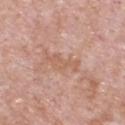Clinical impression: The lesion was tiled from a total-body skin photograph and was not biopsied. Context: The lesion's longest dimension is about 4.5 mm. Cropped from a total-body skin-imaging series; the visible field is about 15 mm. The total-body-photography lesion software estimated a lesion area of about 5 mm² and a symmetry-axis asymmetry near 0.5. The software also gave border irregularity of about 7 on a 0–10 scale, a color-variation rating of about 1.5/10, and peripheral color asymmetry of about 0.5. The analysis additionally found a nevus-likeness score of about 0/100 and a lesion-detection confidence of about 100/100. The tile uses white-light illumination. The lesion is on the chest. The subject is a male approximately 55 years of age.